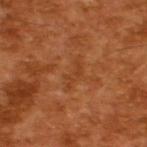<tbp_lesion>
  <biopsy_status>not biopsied; imaged during a skin examination</biopsy_status>
  <automated_metrics>
    <cielab_L>40</cielab_L>
    <cielab_a>26</cielab_a>
    <cielab_b>38</cielab_b>
    <vs_skin_darker_L>5.0</vs_skin_darker_L>
    <vs_skin_contrast_norm>4.5</vs_skin_contrast_norm>
    <border_irregularity_0_10>5.5</border_irregularity_0_10>
    <color_variation_0_10>0.0</color_variation_0_10>
    <peripheral_color_asymmetry>0.0</peripheral_color_asymmetry>
    <lesion_detection_confidence_0_100>95</lesion_detection_confidence_0_100>
  </automated_metrics>
  <image>
    <source>total-body photography crop</source>
    <field_of_view_mm>15</field_of_view_mm>
  </image>
  <patient>
    <sex>male</sex>
    <age_approx>65</age_approx>
  </patient>
</tbp_lesion>